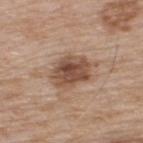Recorded during total-body skin imaging; not selected for excision or biopsy. The tile uses white-light illumination. The lesion-visualizer software estimated a lesion–skin lightness drop of about 13 and a normalized lesion–skin contrast near 9. The analysis additionally found an automated nevus-likeness rating near 75 out of 100. A lesion tile, about 15 mm wide, cut from a 3D total-body photograph. The lesion is on the upper back. The subject is a male approximately 65 years of age. Approximately 5 mm at its widest.